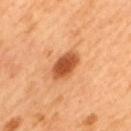<case>
  <biopsy_status>not biopsied; imaged during a skin examination</biopsy_status>
</case>